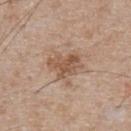Q: Was a biopsy performed?
A: catalogued during a skin exam; not biopsied
Q: What are the patient's age and sex?
A: male, roughly 65 years of age
Q: What did automated image analysis measure?
A: an average lesion color of about L≈54 a*≈18 b*≈30 (CIELAB), roughly 10 lightness units darker than nearby skin, and a normalized border contrast of about 7.5; a lesion-detection confidence of about 100/100
Q: What is the imaging modality?
A: ~15 mm crop, total-body skin-cancer survey
Q: How was the tile lit?
A: white-light illumination
Q: Where on the body is the lesion?
A: the chest